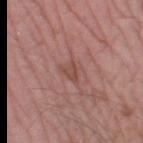Clinical impression: This lesion was catalogued during total-body skin photography and was not selected for biopsy. Background: A close-up tile cropped from a whole-body skin photograph, about 15 mm across. The tile uses white-light illumination. Located on the leg. Longest diameter approximately 2.5 mm. A male subject aged 53–57.